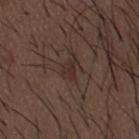Q: Is there a histopathology result?
A: total-body-photography surveillance lesion; no biopsy
Q: How was this image acquired?
A: 15 mm crop, total-body photography
Q: Lesion location?
A: the mid back
Q: Patient demographics?
A: male, aged 48–52